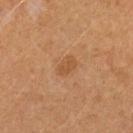Recorded during total-body skin imaging; not selected for excision or biopsy. The lesion is located on the left forearm. A female subject, aged 33–37. A lesion tile, about 15 mm wide, cut from a 3D total-body photograph.